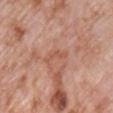The lesion was tiled from a total-body skin photograph and was not biopsied.
The lesion-visualizer software estimated a border-irregularity index near 8/10, a color-variation rating of about 0/10, and peripheral color asymmetry of about 0. It also reported an automated nevus-likeness rating near 0 out of 100 and a detector confidence of about 100 out of 100 that the crop contains a lesion.
A male subject in their 70s.
A region of skin cropped from a whole-body photographic capture, roughly 15 mm wide.
From the chest.